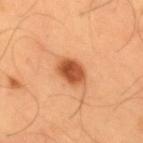Findings:
- follow-up — catalogued during a skin exam; not biopsied
- anatomic site — the mid back
- subject — male, in their mid-50s
- illumination — cross-polarized illumination
- size — about 4 mm
- image source — 15 mm crop, total-body photography